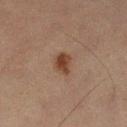follow-up: imaged on a skin check; not biopsied
acquisition: 15 mm crop, total-body photography
subject: female, approximately 60 years of age
anatomic site: the left lower leg